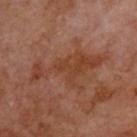Clinical impression:
The lesion was tiled from a total-body skin photograph and was not biopsied.
Context:
Automated tile analysis of the lesion measured a border-irregularity rating of about 9/10, a within-lesion color-variation index near 2.5/10, and radial color variation of about 0.5. It also reported lesion-presence confidence of about 95/100. Cropped from a total-body skin-imaging series; the visible field is about 15 mm. From the upper back. Imaged with cross-polarized lighting. A male patient aged around 70.